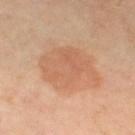Acquisition and patient details:
Located on the right thigh. The patient is a female aged 48 to 52. A 15 mm close-up tile from a total-body photography series done for melanoma screening.